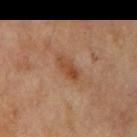Impression:
Captured during whole-body skin photography for melanoma surveillance; the lesion was not biopsied.
Background:
From the right upper arm. Automated image analysis of the tile measured a footprint of about 4 mm² and a shape eccentricity near 0.9. It also reported an average lesion color of about L≈45 a*≈22 b*≈34 (CIELAB), roughly 9 lightness units darker than nearby skin, and a normalized border contrast of about 7.5. The software also gave a border-irregularity index near 3.5/10, internal color variation of about 3.5 on a 0–10 scale, and a peripheral color-asymmetry measure near 1. Captured under cross-polarized illumination. The lesion's longest dimension is about 3.5 mm. Cropped from a whole-body photographic skin survey; the tile spans about 15 mm. A male subject aged approximately 70.